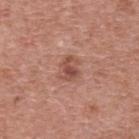Imaged during a routine full-body skin examination; the lesion was not biopsied and no histopathology is available.
A 15 mm close-up extracted from a 3D total-body photography capture.
The patient is a female aged 68 to 72.
The lesion is on the upper back.